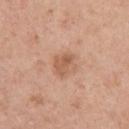Q: Is there a histopathology result?
A: imaged on a skin check; not biopsied
Q: Lesion size?
A: about 3 mm
Q: What are the patient's age and sex?
A: male, aged around 60
Q: What kind of image is this?
A: ~15 mm tile from a whole-body skin photo
Q: Lesion location?
A: the chest
Q: Illumination type?
A: white-light